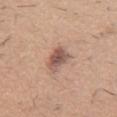notes: no biopsy performed (imaged during a skin exam)
subject: male, aged around 60
automated metrics: a footprint of about 7 mm², an outline eccentricity of about 0.65 (0 = round, 1 = elongated), and a symmetry-axis asymmetry near 0.25; a border-irregularity index near 2.5/10, a within-lesion color-variation index near 4/10, and peripheral color asymmetry of about 1; a nevus-likeness score of about 75/100
image source: 15 mm crop, total-body photography
lesion diameter: ≈3.5 mm
site: the mid back
tile lighting: white-light illumination